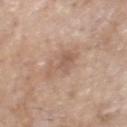Q: Was a biopsy performed?
A: total-body-photography surveillance lesion; no biopsy
Q: Lesion size?
A: ~4 mm (longest diameter)
Q: What is the imaging modality?
A: ~15 mm tile from a whole-body skin photo
Q: Automated lesion metrics?
A: an average lesion color of about L≈57 a*≈18 b*≈28 (CIELAB), about 8 CIELAB-L* units darker than the surrounding skin, and a normalized lesion–skin contrast near 6; a border-irregularity index near 6.5/10, a within-lesion color-variation index near 2.5/10, and radial color variation of about 0.5
Q: Lesion location?
A: the head or neck
Q: What are the patient's age and sex?
A: male, approximately 70 years of age
Q: What lighting was used for the tile?
A: white-light illumination